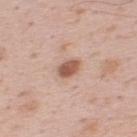Clinical summary: A male subject approximately 35 years of age. The lesion is located on the upper back. Cropped from a whole-body photographic skin survey; the tile spans about 15 mm.